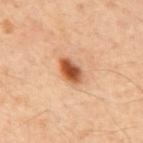{"biopsy_status": "not biopsied; imaged during a skin examination", "patient": {"sex": "male", "age_approx": 60}, "image": {"source": "total-body photography crop", "field_of_view_mm": 15}, "lighting": "cross-polarized", "site": "mid back"}